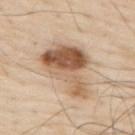Context: The lesion's longest dimension is about 7.5 mm. A 15 mm close-up tile from a total-body photography series done for melanoma screening. The patient is a male aged 78 to 82. The lesion is located on the upper back. The tile uses white-light illumination. An algorithmic analysis of the crop reported border irregularity of about 4.5 on a 0–10 scale and a within-lesion color-variation index near 10/10. And it measured a classifier nevus-likeness of about 95/100 and lesion-presence confidence of about 100/100.